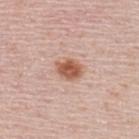Recorded during total-body skin imaging; not selected for excision or biopsy. A roughly 15 mm field-of-view crop from a total-body skin photograph. A male subject in their 50s. The lesion is located on the upper back.